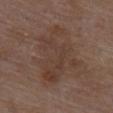This lesion was catalogued during total-body skin photography and was not selected for biopsy. Located on the upper back. The patient is a female aged around 70. Longest diameter approximately 7.5 mm. Imaged with white-light lighting. Automated tile analysis of the lesion measured a footprint of about 26 mm², a shape eccentricity near 0.7, and a shape-asymmetry score of about 0.5 (0 = symmetric). It also reported a mean CIELAB color near L≈38 a*≈16 b*≈24, roughly 6 lightness units darker than nearby skin, and a lesion-to-skin contrast of about 5.5 (normalized; higher = more distinct). A lesion tile, about 15 mm wide, cut from a 3D total-body photograph.